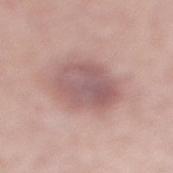{"biopsy_status": "not biopsied; imaged during a skin examination", "patient": {"sex": "male", "age_approx": 65}, "image": {"source": "total-body photography crop", "field_of_view_mm": 15}, "site": "leg"}